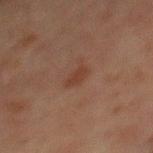Acquisition and patient details: The tile uses cross-polarized illumination. Automated tile analysis of the lesion measured internal color variation of about 0 on a 0–10 scale and a peripheral color-asymmetry measure near 0. The analysis additionally found a classifier nevus-likeness of about 35/100 and lesion-presence confidence of about 100/100. From the abdomen. Measured at roughly 2.5 mm in maximum diameter. A roughly 15 mm field-of-view crop from a total-body skin photograph. The subject is a male in their mid-60s.